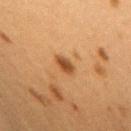The lesion was tiled from a total-body skin photograph and was not biopsied. This is a cross-polarized tile. Approximately 2.5 mm at its widest. The patient is a female aged approximately 40. A roughly 15 mm field-of-view crop from a total-body skin photograph. Located on the upper back.